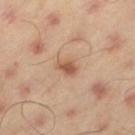notes: total-body-photography surveillance lesion; no biopsy | subject: male, in their mid- to late 40s | image: total-body-photography crop, ~15 mm field of view | lesion size: ~2.5 mm (longest diameter) | TBP lesion metrics: an eccentricity of roughly 0.75 and a symmetry-axis asymmetry near 0.3; a border-irregularity index near 2.5/10 and peripheral color asymmetry of about 1 | anatomic site: the left thigh | lighting: cross-polarized illumination.